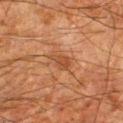Q: Is there a histopathology result?
A: catalogued during a skin exam; not biopsied
Q: Who is the patient?
A: male, approximately 80 years of age
Q: What kind of image is this?
A: total-body-photography crop, ~15 mm field of view
Q: What is the anatomic site?
A: the leg
Q: What did automated image analysis measure?
A: a lesion color around L≈37 a*≈21 b*≈31 in CIELAB, roughly 7 lightness units darker than nearby skin, and a normalized border contrast of about 6
Q: How large is the lesion?
A: ≈3 mm
Q: How was the tile lit?
A: cross-polarized